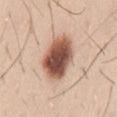The lesion was tiled from a total-body skin photograph and was not biopsied. Measured at roughly 5.5 mm in maximum diameter. A 15 mm close-up tile from a total-body photography series done for melanoma screening. The lesion-visualizer software estimated an average lesion color of about L≈54 a*≈21 b*≈28 (CIELAB), about 21 CIELAB-L* units darker than the surrounding skin, and a normalized border contrast of about 13. It also reported a color-variation rating of about 8/10 and peripheral color asymmetry of about 2. Located on the lower back. A male patient, aged 38 to 42.